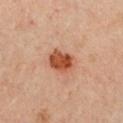Findings:
– tile lighting · cross-polarized illumination
– patient · female, aged 33–37
– location · the chest
– TBP lesion metrics · an area of roughly 7.5 mm², a shape eccentricity near 0.65, and a shape-asymmetry score of about 0.15 (0 = symmetric); a classifier nevus-likeness of about 100/100 and lesion-presence confidence of about 100/100
– lesion diameter · about 3.5 mm
– image source · ~15 mm crop, total-body skin-cancer survey